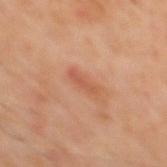Imaged during a routine full-body skin examination; the lesion was not biopsied and no histopathology is available. The patient is a male about 60 years old. Approximately 3.5 mm at its widest. The lesion-visualizer software estimated an outline eccentricity of about 0.95 (0 = round, 1 = elongated). The analysis additionally found a border-irregularity rating of about 3.5/10, a within-lesion color-variation index near 0/10, and peripheral color asymmetry of about 0. The lesion is located on the mid back. A close-up tile cropped from a whole-body skin photograph, about 15 mm across.